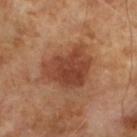Q: Was a biopsy performed?
A: total-body-photography surveillance lesion; no biopsy
Q: What lighting was used for the tile?
A: cross-polarized illumination
Q: What is the imaging modality?
A: ~15 mm tile from a whole-body skin photo
Q: Automated lesion metrics?
A: a lesion–skin lightness drop of about 11 and a normalized lesion–skin contrast near 8.5; a nevus-likeness score of about 70/100 and lesion-presence confidence of about 100/100
Q: How large is the lesion?
A: about 5.5 mm
Q: What are the patient's age and sex?
A: male, in their mid- to late 60s